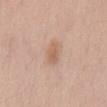{
  "biopsy_status": "not biopsied; imaged during a skin examination",
  "lesion_size": {
    "long_diameter_mm_approx": 3.0
  },
  "patient": {
    "sex": "female",
    "age_approx": 35
  },
  "image": {
    "source": "total-body photography crop",
    "field_of_view_mm": 15
  },
  "automated_metrics": {
    "area_mm2_approx": 4.5,
    "eccentricity": 0.8,
    "shape_asymmetry": 0.25,
    "cielab_L": 61,
    "cielab_a": 19,
    "cielab_b": 30,
    "vs_skin_darker_L": 8.0,
    "border_irregularity_0_10": 2.5,
    "color_variation_0_10": 2.0,
    "peripheral_color_asymmetry": 0.5
  },
  "site": "chest",
  "lighting": "white-light"
}